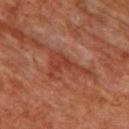Assessment: This lesion was catalogued during total-body skin photography and was not selected for biopsy. Clinical summary: A lesion tile, about 15 mm wide, cut from a 3D total-body photograph. The lesion is on the front of the torso. Imaged with cross-polarized lighting. About 5.5 mm across. The total-body-photography lesion software estimated an eccentricity of roughly 0.85. The software also gave a lesion color around L≈36 a*≈26 b*≈29 in CIELAB and about 7 CIELAB-L* units darker than the surrounding skin. And it measured a border-irregularity rating of about 9/10 and a peripheral color-asymmetry measure near 1. The software also gave lesion-presence confidence of about 60/100. A male subject, aged 58 to 62.